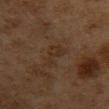notes = catalogued during a skin exam; not biopsied | image source = ~15 mm tile from a whole-body skin photo | subject = female, aged around 60 | image-analysis metrics = a mean CIELAB color near L≈26 a*≈14 b*≈23, a lesion–skin lightness drop of about 4, and a normalized lesion–skin contrast near 5; an automated nevus-likeness rating near 0 out of 100 and a lesion-detection confidence of about 90/100 | illumination = cross-polarized illumination | location = the chest | lesion size = ≈4.5 mm.